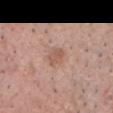Clinical impression: Imaged during a routine full-body skin examination; the lesion was not biopsied and no histopathology is available. Background: Cropped from a total-body skin-imaging series; the visible field is about 15 mm. The lesion is located on the head or neck. A male subject, aged approximately 55. Longest diameter approximately 3 mm. This is a white-light tile.